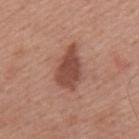The lesion is located on the mid back. Approximately 5.5 mm at its widest. The tile uses white-light illumination. A close-up tile cropped from a whole-body skin photograph, about 15 mm across. A male patient, aged approximately 65.